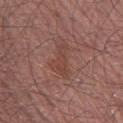Recorded during total-body skin imaging; not selected for excision or biopsy.
This is a white-light tile.
The total-body-photography lesion software estimated an area of roughly 7 mm², an eccentricity of roughly 0.85, and two-axis asymmetry of about 0.45. The analysis additionally found border irregularity of about 5 on a 0–10 scale and a peripheral color-asymmetry measure near 0.5.
A close-up tile cropped from a whole-body skin photograph, about 15 mm across.
A male subject aged 53 to 57.
On the leg.